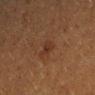Findings:
* follow-up: no biopsy performed (imaged during a skin exam)
* imaging modality: 15 mm crop, total-body photography
* subject: female, aged approximately 40
* illumination: cross-polarized
* automated lesion analysis: a mean CIELAB color near L≈26 a*≈18 b*≈24, a lesion–skin lightness drop of about 5, and a normalized border contrast of about 6; a border-irregularity rating of about 3/10, internal color variation of about 2 on a 0–10 scale, and radial color variation of about 1; a lesion-detection confidence of about 100/100
* body site: the left forearm
* lesion diameter: ≈2 mm